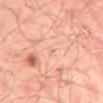{"biopsy_status": "not biopsied; imaged during a skin examination", "site": "back", "patient": {"sex": "male", "age_approx": 50}, "image": {"source": "total-body photography crop", "field_of_view_mm": 15}, "lighting": "cross-polarized"}